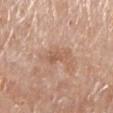The lesion was photographed on a routine skin check and not biopsied; there is no pathology result. A female subject roughly 60 years of age. The lesion is on the left lower leg. Cropped from a whole-body photographic skin survey; the tile spans about 15 mm. Measured at roughly 3.5 mm in maximum diameter. The lesion-visualizer software estimated a footprint of about 4 mm² and an eccentricity of roughly 0.9. The analysis additionally found a lesion color around L≈57 a*≈20 b*≈31 in CIELAB, roughly 8 lightness units darker than nearby skin, and a normalized lesion–skin contrast near 6. It also reported a border-irregularity rating of about 4/10 and peripheral color asymmetry of about 0.